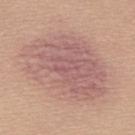Q: Was a biopsy performed?
A: no biopsy performed (imaged during a skin exam)
Q: What are the patient's age and sex?
A: female, roughly 25 years of age
Q: Lesion location?
A: the upper back
Q: What is the imaging modality?
A: 15 mm crop, total-body photography